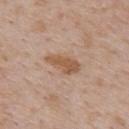• image — ~15 mm crop, total-body skin-cancer survey
• subject — male, aged around 60
• lesion diameter — ~4 mm (longest diameter)
• automated lesion analysis — an average lesion color of about L≈55 a*≈19 b*≈32 (CIELAB) and about 10 CIELAB-L* units darker than the surrounding skin; a border-irregularity index near 3/10; a classifier nevus-likeness of about 75/100 and a detector confidence of about 100 out of 100 that the crop contains a lesion
• site — the upper back